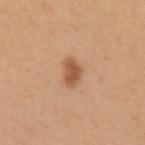The lesion was tiled from a total-body skin photograph and was not biopsied. Cropped from a whole-body photographic skin survey; the tile spans about 15 mm. Imaged with white-light lighting. The patient is a female aged 43 to 47. Automated image analysis of the tile measured a border-irregularity index near 2.5/10 and radial color variation of about 0.5. From the left upper arm. The lesion's longest dimension is about 3 mm.